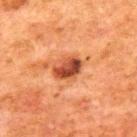<record>
<biopsy_status>not biopsied; imaged during a skin examination</biopsy_status>
<patient>
  <sex>male</sex>
  <age_approx>80</age_approx>
</patient>
<lighting>cross-polarized</lighting>
<image>
  <source>total-body photography crop</source>
  <field_of_view_mm>15</field_of_view_mm>
</image>
<site>back</site>
<lesion_size>
  <long_diameter_mm_approx>3.5</long_diameter_mm_approx>
</lesion_size>
<automated_metrics>
  <cielab_L>39</cielab_L>
  <cielab_a>26</cielab_a>
  <cielab_b>32</cielab_b>
  <vs_skin_darker_L>14.0</vs_skin_darker_L>
  <vs_skin_contrast_norm>11.0</vs_skin_contrast_norm>
  <border_irregularity_0_10>2.0</border_irregularity_0_10>
  <color_variation_0_10>6.5</color_variation_0_10>
  <nevus_likeness_0_100>90</nevus_likeness_0_100>
</automated_metrics>
</record>